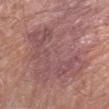Notes:
– follow-up — no biopsy performed (imaged during a skin exam)
– patient — male, aged around 80
– site — the arm
– lesion size — ≈10 mm
– image — ~15 mm tile from a whole-body skin photo
– automated metrics — a lesion color around L≈52 a*≈22 b*≈20 in CIELAB and a normalized border contrast of about 5.5; border irregularity of about 5 on a 0–10 scale, internal color variation of about 5 on a 0–10 scale, and radial color variation of about 1.5; an automated nevus-likeness rating near 0 out of 100 and a detector confidence of about 90 out of 100 that the crop contains a lesion